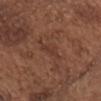The lesion was tiled from a total-body skin photograph and was not biopsied. A male subject aged approximately 75. Located on the chest. Approximately 3 mm at its widest. The total-body-photography lesion software estimated an automated nevus-likeness rating near 0 out of 100 and lesion-presence confidence of about 90/100. Captured under white-light illumination. This image is a 15 mm lesion crop taken from a total-body photograph.